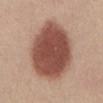Assessment: The lesion was photographed on a routine skin check and not biopsied; there is no pathology result. Context: A roughly 15 mm field-of-view crop from a total-body skin photograph. The subject is a female about 40 years old. The lesion is located on the abdomen.